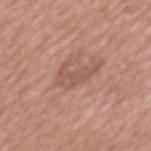{"biopsy_status": "not biopsied; imaged during a skin examination", "patient": {"sex": "male", "age_approx": 45}, "image": {"source": "total-body photography crop", "field_of_view_mm": 15}, "site": "chest"}